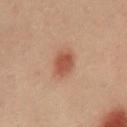<lesion>
<biopsy_status>not biopsied; imaged during a skin examination</biopsy_status>
<patient>
  <sex>male</sex>
  <age_approx>40</age_approx>
</patient>
<site>front of the torso</site>
<image>
  <source>total-body photography crop</source>
  <field_of_view_mm>15</field_of_view_mm>
</image>
</lesion>